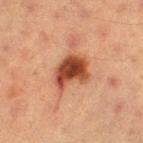Cropped from a total-body skin-imaging series; the visible field is about 15 mm. From the left thigh. Measured at roughly 4.5 mm in maximum diameter. A female patient aged around 55.